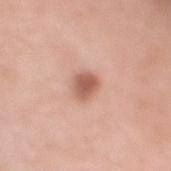Part of a total-body skin-imaging series; this lesion was reviewed on a skin check and was not flagged for biopsy. A female subject approximately 70 years of age. A roughly 15 mm field-of-view crop from a total-body skin photograph. Imaged with white-light lighting. An algorithmic analysis of the crop reported an area of roughly 5 mm², a shape eccentricity near 0.35, and a symmetry-axis asymmetry near 0.2. And it measured a border-irregularity rating of about 2/10, a within-lesion color-variation index near 3/10, and a peripheral color-asymmetry measure near 1. It also reported an automated nevus-likeness rating near 95 out of 100. The recorded lesion diameter is about 2.5 mm. From the mid back.